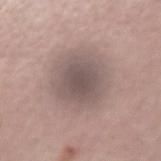Recorded during total-body skin imaging; not selected for excision or biopsy. On the right forearm. About 6.5 mm across. Imaged with white-light lighting. A male patient in their 60s. A 15 mm close-up extracted from a 3D total-body photography capture.